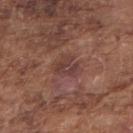Q: Is there a histopathology result?
A: total-body-photography surveillance lesion; no biopsy
Q: What lighting was used for the tile?
A: white-light illumination
Q: Who is the patient?
A: male, approximately 75 years of age
Q: What is the anatomic site?
A: the right upper arm
Q: What is the lesion's diameter?
A: ≈3.5 mm
Q: What is the imaging modality?
A: total-body-photography crop, ~15 mm field of view